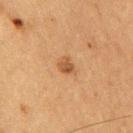The lesion was photographed on a routine skin check and not biopsied; there is no pathology result. Captured under cross-polarized illumination. Cropped from a total-body skin-imaging series; the visible field is about 15 mm. The subject is a male roughly 65 years of age. The lesion's longest dimension is about 2.5 mm. Located on the chest.